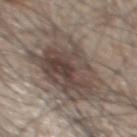body site: the mid back
imaging modality: ~15 mm crop, total-body skin-cancer survey
patient: male, aged around 45